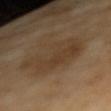notes = catalogued during a skin exam; not biopsied | body site = the right forearm | patient = female, aged approximately 70 | image = total-body-photography crop, ~15 mm field of view.